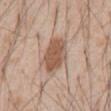illumination — white-light | anatomic site — the abdomen | subject — male, in their mid-50s | image-analysis metrics — an average lesion color of about L≈55 a*≈19 b*≈29 (CIELAB), about 12 CIELAB-L* units darker than the surrounding skin, and a lesion-to-skin contrast of about 8.5 (normalized; higher = more distinct); a border-irregularity index near 2/10; a nevus-likeness score of about 90/100 and lesion-presence confidence of about 100/100 | acquisition — 15 mm crop, total-body photography | lesion diameter — ≈5 mm.